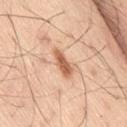Impression:
The lesion was photographed on a routine skin check and not biopsied; there is no pathology result.
Clinical summary:
A lesion tile, about 15 mm wide, cut from a 3D total-body photograph. Captured under white-light illumination. Located on the right thigh. The patient is a male approximately 60 years of age. Approximately 4 mm at its widest. The lesion-visualizer software estimated a footprint of about 5.5 mm², a shape eccentricity near 0.9, and two-axis asymmetry of about 0.2. The analysis additionally found a border-irregularity rating of about 2.5/10, internal color variation of about 3 on a 0–10 scale, and a peripheral color-asymmetry measure near 1.